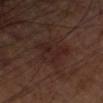Context:
A lesion tile, about 15 mm wide, cut from a 3D total-body photograph. The patient is a male in their mid-60s. From the left forearm. Captured under cross-polarized illumination.